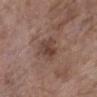| key | value |
|---|---|
| notes | catalogued during a skin exam; not biopsied |
| subject | female, about 85 years old or older |
| illumination | white-light |
| automated metrics | a lesion area of about 6.5 mm², a shape eccentricity near 0.65, and two-axis asymmetry of about 0.25; a mean CIELAB color near L≈42 a*≈18 b*≈23 and a normalized border contrast of about 7; border irregularity of about 2.5 on a 0–10 scale and a within-lesion color-variation index near 4.5/10; a nevus-likeness score of about 45/100 and a detector confidence of about 100 out of 100 that the crop contains a lesion |
| diameter | about 3 mm |
| image source | ~15 mm tile from a whole-body skin photo |
| location | the left lower leg |